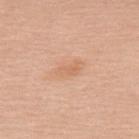biopsy status=total-body-photography surveillance lesion; no biopsy
image source=15 mm crop, total-body photography
anatomic site=the upper back
subject=female, roughly 55 years of age